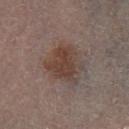The patient is a female aged around 80. Captured under cross-polarized illumination. The lesion is on the right leg. The recorded lesion diameter is about 5 mm. A 15 mm close-up tile from a total-body photography series done for melanoma screening.